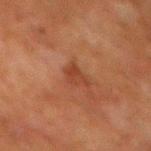Findings:
– workup — no biopsy performed (imaged during a skin exam)
– patient — male, approximately 80 years of age
– site — the back
– image source — total-body-photography crop, ~15 mm field of view
– lesion diameter — ≈3 mm